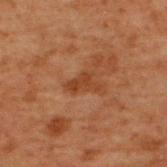  biopsy_status: not biopsied; imaged during a skin examination
  lesion_size:
    long_diameter_mm_approx: 3.5
  patient:
    sex: male
    age_approx: 70
  lighting: cross-polarized
  image:
    source: total-body photography crop
    field_of_view_mm: 15
  site: upper back